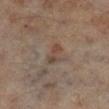Case summary:
* biopsy status — no biopsy performed (imaged during a skin exam)
* TBP lesion metrics — a lesion color around L≈42 a*≈15 b*≈24 in CIELAB, a lesion–skin lightness drop of about 7, and a lesion-to-skin contrast of about 6 (normalized; higher = more distinct); a nevus-likeness score of about 5/100 and lesion-presence confidence of about 100/100
* location — the right lower leg
* lighting — cross-polarized
* patient — male, aged 63 to 67
* diameter — ~3 mm (longest diameter)
* acquisition — 15 mm crop, total-body photography